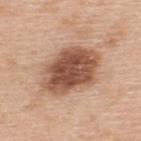follow-up=imaged on a skin check; not biopsied
subject=male, aged around 50
acquisition=total-body-photography crop, ~15 mm field of view
anatomic site=the back